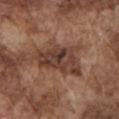<record>
  <biopsy_status>not biopsied; imaged during a skin examination</biopsy_status>
  <automated_metrics>
    <vs_skin_darker_L>11.0</vs_skin_darker_L>
    <vs_skin_contrast_norm>9.5</vs_skin_contrast_norm>
    <color_variation_0_10>6.5</color_variation_0_10>
    <peripheral_color_asymmetry>2.5</peripheral_color_asymmetry>
  </automated_metrics>
  <image>
    <source>total-body photography crop</source>
    <field_of_view_mm>15</field_of_view_mm>
  </image>
  <patient>
    <sex>male</sex>
    <age_approx>75</age_approx>
  </patient>
  <lighting>white-light</lighting>
  <site>front of the torso</site>
</record>